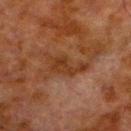| key | value |
|---|---|
| follow-up | imaged on a skin check; not biopsied |
| location | the left lower leg |
| TBP lesion metrics | two-axis asymmetry of about 0.4; an average lesion color of about L≈29 a*≈19 b*≈28 (CIELAB), roughly 6 lightness units darker than nearby skin, and a normalized lesion–skin contrast near 7 |
| subject | male, in their 80s |
| size | ~5 mm (longest diameter) |
| tile lighting | cross-polarized |
| image | ~15 mm crop, total-body skin-cancer survey |